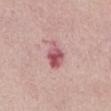Clinical impression:
This lesion was catalogued during total-body skin photography and was not selected for biopsy.
Image and clinical context:
Captured under white-light illumination. Automated tile analysis of the lesion measured a lesion area of about 6.5 mm² and an outline eccentricity of about 0.75 (0 = round, 1 = elongated). It also reported a lesion-to-skin contrast of about 9 (normalized; higher = more distinct). A female subject, approximately 70 years of age. A lesion tile, about 15 mm wide, cut from a 3D total-body photograph. Located on the front of the torso.